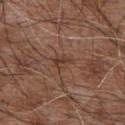Clinical summary: A region of skin cropped from a whole-body photographic capture, roughly 15 mm wide. The subject is a male about 75 years old. On the abdomen. Automated tile analysis of the lesion measured a lesion color around L≈39 a*≈19 b*≈26 in CIELAB, about 7 CIELAB-L* units darker than the surrounding skin, and a normalized lesion–skin contrast near 6. It also reported a border-irregularity index near 3/10 and peripheral color asymmetry of about 1.5. This is a white-light tile. Longest diameter approximately 2.5 mm.